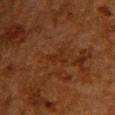tile lighting — cross-polarized; automated lesion analysis — an automated nevus-likeness rating near 0 out of 100 and a detector confidence of about 65 out of 100 that the crop contains a lesion; patient — female, roughly 50 years of age; size — ~3 mm (longest diameter); imaging modality — 15 mm crop, total-body photography; site — the back.